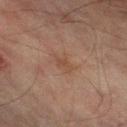| field | value |
|---|---|
| follow-up | imaged on a skin check; not biopsied |
| lesion size | ≈3 mm |
| illumination | cross-polarized |
| image | ~15 mm tile from a whole-body skin photo |
| patient | male, approximately 75 years of age |
| anatomic site | the right thigh |